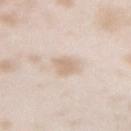This lesion was catalogued during total-body skin photography and was not selected for biopsy.
A female patient, roughly 25 years of age.
Measured at roughly 2.5 mm in maximum diameter.
Located on the right forearm.
Automated tile analysis of the lesion measured an area of roughly 4 mm², an eccentricity of roughly 0.7, and two-axis asymmetry of about 0.25. And it measured about 8 CIELAB-L* units darker than the surrounding skin. And it measured a nevus-likeness score of about 5/100 and a lesion-detection confidence of about 95/100.
Captured under white-light illumination.
Cropped from a total-body skin-imaging series; the visible field is about 15 mm.